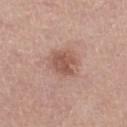Q: Was a biopsy performed?
A: imaged on a skin check; not biopsied
Q: Patient demographics?
A: female, aged 63 to 67
Q: What kind of image is this?
A: ~15 mm crop, total-body skin-cancer survey
Q: What is the anatomic site?
A: the right thigh
Q: What is the lesion's diameter?
A: ~3 mm (longest diameter)